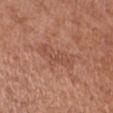Q: Was this lesion biopsied?
A: catalogued during a skin exam; not biopsied
Q: What did automated image analysis measure?
A: border irregularity of about 4 on a 0–10 scale, internal color variation of about 2.5 on a 0–10 scale, and peripheral color asymmetry of about 1
Q: What is the anatomic site?
A: the left arm
Q: What is the lesion's diameter?
A: ≈5 mm
Q: What is the imaging modality?
A: total-body-photography crop, ~15 mm field of view
Q: What are the patient's age and sex?
A: female, aged 63 to 67